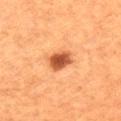A male patient, approximately 60 years of age. The recorded lesion diameter is about 3 mm. A 15 mm crop from a total-body photograph taken for skin-cancer surveillance. The lesion is on the leg.